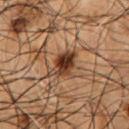Findings:
• site · the front of the torso
• image · ~15 mm crop, total-body skin-cancer survey
• patient · male, in their mid-50s